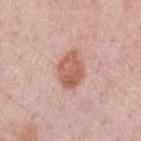The lesion was tiled from a total-body skin photograph and was not biopsied. A 15 mm close-up extracted from a 3D total-body photography capture. Captured under white-light illumination. A female patient, approximately 30 years of age. Measured at roughly 4 mm in maximum diameter. The lesion is on the left upper arm.